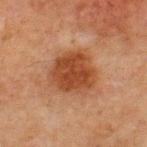No biopsy was performed on this lesion — it was imaged during a full skin examination and was not determined to be concerning. The subject is a male approximately 65 years of age. A lesion tile, about 15 mm wide, cut from a 3D total-body photograph. Located on the chest.